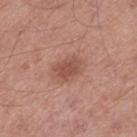Q: Was a biopsy performed?
A: total-body-photography surveillance lesion; no biopsy
Q: What are the patient's age and sex?
A: male, aged 63 to 67
Q: Where on the body is the lesion?
A: the left thigh
Q: How was this image acquired?
A: 15 mm crop, total-body photography